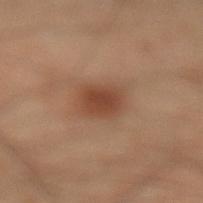biopsy status: total-body-photography surveillance lesion; no biopsy
patient: male, in their 50s
anatomic site: the left lower leg
image: ~15 mm crop, total-body skin-cancer survey